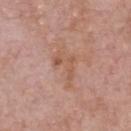Findings:
• workup — no biopsy performed (imaged during a skin exam)